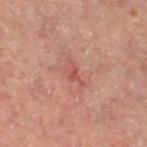Assessment:
Recorded during total-body skin imaging; not selected for excision or biopsy.
Acquisition and patient details:
A female subject approximately 60 years of age. The lesion is located on the right thigh. A region of skin cropped from a whole-body photographic capture, roughly 15 mm wide. Imaged with cross-polarized lighting. Longest diameter approximately 3 mm.